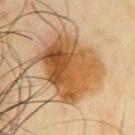Imaged during a routine full-body skin examination; the lesion was not biopsied and no histopathology is available.
The subject is a male about 65 years old.
Measured at roughly 7 mm in maximum diameter.
Captured under cross-polarized illumination.
Located on the chest.
A 15 mm crop from a total-body photograph taken for skin-cancer surveillance.
The total-body-photography lesion software estimated an average lesion color of about L≈55 a*≈22 b*≈42 (CIELAB) and a normalized lesion–skin contrast near 10.5. The analysis additionally found border irregularity of about 3.5 on a 0–10 scale, a color-variation rating of about 9.5/10, and a peripheral color-asymmetry measure near 3.5.